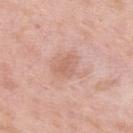| key | value |
|---|---|
| workup | imaged on a skin check; not biopsied |
| tile lighting | white-light illumination |
| automated lesion analysis | a lesion area of about 10 mm², an eccentricity of roughly 0.5, and two-axis asymmetry of about 0.25; a lesion–skin lightness drop of about 7; a border-irregularity rating of about 3.5/10, a within-lesion color-variation index near 3/10, and a peripheral color-asymmetry measure near 1; an automated nevus-likeness rating near 0 out of 100 |
| subject | male, aged approximately 55 |
| imaging modality | 15 mm crop, total-body photography |
| location | the upper back |